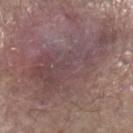The lesion was tiled from a total-body skin photograph and was not biopsied.
A male patient, about 80 years old.
On the leg.
A 15 mm close-up tile from a total-body photography series done for melanoma screening.
Longest diameter approximately 13 mm.
Captured under white-light illumination.